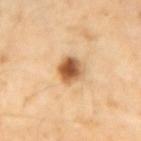Part of a total-body skin-imaging series; this lesion was reviewed on a skin check and was not flagged for biopsy. Located on the left forearm. The patient is a male in their 40s. The recorded lesion diameter is about 3 mm. A roughly 15 mm field-of-view crop from a total-body skin photograph.